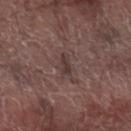Assessment:
Captured during whole-body skin photography for melanoma surveillance; the lesion was not biopsied.
Clinical summary:
Longest diameter approximately 3 mm. This image is a 15 mm lesion crop taken from a total-body photograph. Imaged with white-light lighting. A male patient, about 70 years old. From the left forearm.